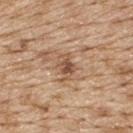Q: Was this lesion biopsied?
A: no biopsy performed (imaged during a skin exam)
Q: Who is the patient?
A: male, aged around 70
Q: What is the imaging modality?
A: ~15 mm tile from a whole-body skin photo
Q: What is the anatomic site?
A: the upper back